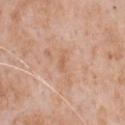workup: total-body-photography surveillance lesion; no biopsy
subject: male, in their mid- to late 60s
imaging modality: total-body-photography crop, ~15 mm field of view
anatomic site: the chest